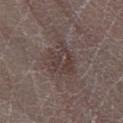Impression: The lesion was tiled from a total-body skin photograph and was not biopsied. Clinical summary: About 4 mm across. The lesion is located on the right lower leg. The patient is a female aged approximately 70. Cropped from a total-body skin-imaging series; the visible field is about 15 mm. The tile uses white-light illumination.